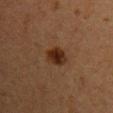No biopsy was performed on this lesion — it was imaged during a full skin examination and was not determined to be concerning. Automated image analysis of the tile measured an outline eccentricity of about 0.55 (0 = round, 1 = elongated) and a shape-asymmetry score of about 0.15 (0 = symmetric). It also reported a mean CIELAB color near L≈23 a*≈17 b*≈24 and a normalized lesion–skin contrast near 10. It also reported an automated nevus-likeness rating near 95 out of 100 and lesion-presence confidence of about 100/100. A region of skin cropped from a whole-body photographic capture, roughly 15 mm wide. A female subject, approximately 40 years of age. Captured under cross-polarized illumination. Located on the arm. Measured at roughly 3 mm in maximum diameter.